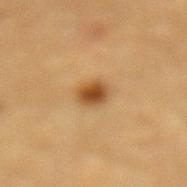notes — no biopsy performed (imaged during a skin exam); lesion size — ~2.5 mm (longest diameter); subject — male, in their mid- to late 80s or older; site — the back; acquisition — total-body-photography crop, ~15 mm field of view.